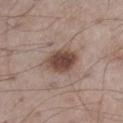Recorded during total-body skin imaging; not selected for excision or biopsy.
The subject is a male roughly 55 years of age.
A lesion tile, about 15 mm wide, cut from a 3D total-body photograph.
From the left thigh.